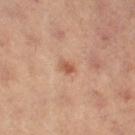Cropped from a total-body skin-imaging series; the visible field is about 15 mm. Longest diameter approximately 2 mm. The lesion is located on the left thigh. This is a cross-polarized tile. An algorithmic analysis of the crop reported a footprint of about 2.5 mm², a shape eccentricity near 0.75, and a symmetry-axis asymmetry near 0.3. The analysis additionally found an automated nevus-likeness rating near 75 out of 100. The patient is a female about 40 years old.